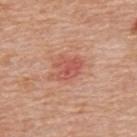This lesion was catalogued during total-body skin photography and was not selected for biopsy. The patient is a male aged approximately 60. A 15 mm close-up tile from a total-body photography series done for melanoma screening. Imaged with white-light lighting. The lesion is on the mid back.